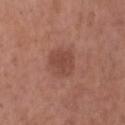| field | value |
|---|---|
| notes | no biopsy performed (imaged during a skin exam) |
| patient | male, aged approximately 35 |
| imaging modality | total-body-photography crop, ~15 mm field of view |
| site | the head or neck |